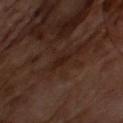Recorded during total-body skin imaging; not selected for excision or biopsy.
Automated tile analysis of the lesion measured a mean CIELAB color near L≈19 a*≈17 b*≈21 and a normalized lesion–skin contrast near 7. The software also gave an automated nevus-likeness rating near 0 out of 100 and lesion-presence confidence of about 60/100.
Approximately 2.5 mm at its widest.
A male subject roughly 65 years of age.
Imaged with cross-polarized lighting.
A roughly 15 mm field-of-view crop from a total-body skin photograph.